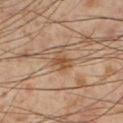biopsy_status: not biopsied; imaged during a skin examination
lesion_size:
  long_diameter_mm_approx: 3.0
site: left lower leg
automated_metrics:
  cielab_L: 51
  cielab_a: 20
  cielab_b: 34
  vs_skin_darker_L: 10.0
  vs_skin_contrast_norm: 7.5
  nevus_likeness_0_100: 5
  lesion_detection_confidence_0_100: 100
image:
  source: total-body photography crop
  field_of_view_mm: 15
lighting: cross-polarized
patient:
  sex: male
  age_approx: 55